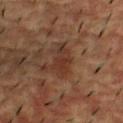Impression: The lesion was tiled from a total-body skin photograph and was not biopsied. Image and clinical context: From the upper back. A male subject, aged around 55. This is a cross-polarized tile. A 15 mm crop from a total-body photograph taken for skin-cancer surveillance.